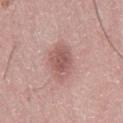No biopsy was performed on this lesion — it was imaged during a full skin examination and was not determined to be concerning.
From the right thigh.
A male patient, in their 50s.
Captured under white-light illumination.
A lesion tile, about 15 mm wide, cut from a 3D total-body photograph.
An algorithmic analysis of the crop reported border irregularity of about 2 on a 0–10 scale and radial color variation of about 1.